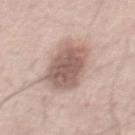Case summary:
– notes: total-body-photography surveillance lesion; no biopsy
– tile lighting: white-light
– site: the abdomen
– subject: male, in their 60s
– imaging modality: total-body-photography crop, ~15 mm field of view
– image-analysis metrics: an area of roughly 20 mm², a shape eccentricity near 0.8, and a symmetry-axis asymmetry near 0.15; an average lesion color of about L≈58 a*≈18 b*≈23 (CIELAB), about 14 CIELAB-L* units darker than the surrounding skin, and a normalized border contrast of about 9